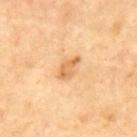Measured at roughly 3.5 mm in maximum diameter. An algorithmic analysis of the crop reported an eccentricity of roughly 0.85 and two-axis asymmetry of about 0.35. The software also gave an average lesion color of about L≈67 a*≈22 b*≈44 (CIELAB), about 10 CIELAB-L* units darker than the surrounding skin, and a normalized border contrast of about 6.5. A 15 mm close-up extracted from a 3D total-body photography capture. On the mid back. The patient is a male aged approximately 70. The tile uses cross-polarized illumination.